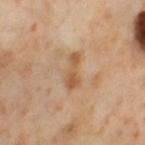Case summary:
* notes · no biopsy performed (imaged during a skin exam)
* subject · female, aged around 55
* site · the leg
* diameter · ~4 mm (longest diameter)
* image source · ~15 mm crop, total-body skin-cancer survey